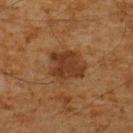Clinical impression: Imaged during a routine full-body skin examination; the lesion was not biopsied and no histopathology is available. Acquisition and patient details: This image is a 15 mm lesion crop taken from a total-body photograph. From the upper back. The recorded lesion diameter is about 4 mm. The patient is a male aged around 60.